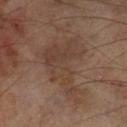Part of a total-body skin-imaging series; this lesion was reviewed on a skin check and was not flagged for biopsy. Imaged with cross-polarized lighting. An algorithmic analysis of the crop reported border irregularity of about 9 on a 0–10 scale, a color-variation rating of about 3.5/10, and peripheral color asymmetry of about 1. The analysis additionally found a lesion-detection confidence of about 95/100. A 15 mm crop from a total-body photograph taken for skin-cancer surveillance. The lesion is located on the left lower leg. A male subject aged 68–72.